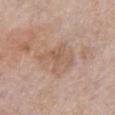Q: Was a biopsy performed?
A: imaged on a skin check; not biopsied
Q: Automated lesion metrics?
A: a footprint of about 7 mm², a shape eccentricity near 0.8, and two-axis asymmetry of about 0.55; a lesion color around L≈57 a*≈18 b*≈29 in CIELAB, roughly 7 lightness units darker than nearby skin, and a normalized border contrast of about 5.5; a nevus-likeness score of about 0/100 and lesion-presence confidence of about 100/100
Q: How was the tile lit?
A: white-light illumination
Q: Lesion size?
A: ~4.5 mm (longest diameter)
Q: How was this image acquired?
A: 15 mm crop, total-body photography
Q: Patient demographics?
A: female, aged 63 to 67
Q: Lesion location?
A: the chest